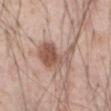Part of a total-body skin-imaging series; this lesion was reviewed on a skin check and was not flagged for biopsy.
A male patient aged 53–57.
A 15 mm crop from a total-body photograph taken for skin-cancer surveillance.
On the abdomen.